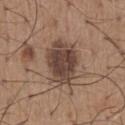The lesion was tiled from a total-body skin photograph and was not biopsied. The total-body-photography lesion software estimated a lesion area of about 18 mm² and an outline eccentricity of about 0.7 (0 = round, 1 = elongated). The software also gave a border-irregularity rating of about 2/10, internal color variation of about 3.5 on a 0–10 scale, and radial color variation of about 1. Approximately 5.5 mm at its widest. A region of skin cropped from a whole-body photographic capture, roughly 15 mm wide. The lesion is located on the chest. A male patient, about 65 years old. Imaged with white-light lighting.